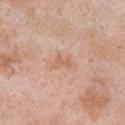{"biopsy_status": "not biopsied; imaged during a skin examination", "lesion_size": {"long_diameter_mm_approx": 3.5}, "patient": {"sex": "male", "age_approx": 55}, "lighting": "white-light", "image": {"source": "total-body photography crop", "field_of_view_mm": 15}, "site": "left upper arm"}